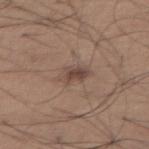Assessment: No biopsy was performed on this lesion — it was imaged during a full skin examination and was not determined to be concerning. Clinical summary: A male patient roughly 65 years of age. Measured at roughly 4 mm in maximum diameter. The lesion is located on the abdomen. The lesion-visualizer software estimated about 9 CIELAB-L* units darker than the surrounding skin and a normalized lesion–skin contrast near 7. This image is a 15 mm lesion crop taken from a total-body photograph.